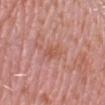Impression: This lesion was catalogued during total-body skin photography and was not selected for biopsy. Context: The recorded lesion diameter is about 3 mm. On the right lower leg. The total-body-photography lesion software estimated about 6 CIELAB-L* units darker than the surrounding skin. And it measured border irregularity of about 3 on a 0–10 scale, a within-lesion color-variation index near 1.5/10, and a peripheral color-asymmetry measure near 0.5. This is a white-light tile. Cropped from a total-body skin-imaging series; the visible field is about 15 mm. The patient is a female in their 40s.